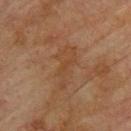workup — total-body-photography surveillance lesion; no biopsy
subject — male, in their mid-70s
lighting — cross-polarized illumination
lesion size — ~5 mm (longest diameter)
location — the back
image source — 15 mm crop, total-body photography